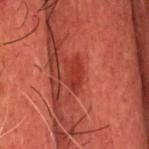The lesion was photographed on a routine skin check and not biopsied; there is no pathology result.